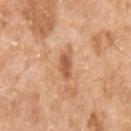{
  "biopsy_status": "not biopsied; imaged during a skin examination",
  "automated_metrics": {
    "cielab_L": 58,
    "cielab_a": 24,
    "cielab_b": 36,
    "vs_skin_darker_L": 11.0,
    "vs_skin_contrast_norm": 7.5
  },
  "lesion_size": {
    "long_diameter_mm_approx": 3.0
  },
  "site": "left upper arm",
  "image": {
    "source": "total-body photography crop",
    "field_of_view_mm": 15
  },
  "patient": {
    "sex": "female",
    "age_approx": 75
  },
  "lighting": "white-light"
}